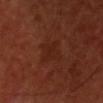Part of a total-body skin-imaging series; this lesion was reviewed on a skin check and was not flagged for biopsy.
This is a cross-polarized tile.
The lesion is on the upper back.
This image is a 15 mm lesion crop taken from a total-body photograph.
The patient is a male aged 48–52.
An algorithmic analysis of the crop reported a lesion area of about 7.5 mm² and two-axis asymmetry of about 0.35.